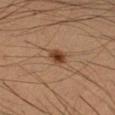Captured during whole-body skin photography for melanoma surveillance; the lesion was not biopsied.
A close-up tile cropped from a whole-body skin photograph, about 15 mm across.
An algorithmic analysis of the crop reported about 11 CIELAB-L* units darker than the surrounding skin and a lesion-to-skin contrast of about 9.5 (normalized; higher = more distinct).
Measured at roughly 3 mm in maximum diameter.
On the arm.
The subject is a male about 35 years old.
The tile uses cross-polarized illumination.